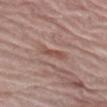Imaged during a routine full-body skin examination; the lesion was not biopsied and no histopathology is available.
On the left thigh.
Automated image analysis of the tile measured a classifier nevus-likeness of about 0/100 and a lesion-detection confidence of about 65/100.
A female patient aged 63 to 67.
The lesion's longest dimension is about 3.5 mm.
The tile uses white-light illumination.
Cropped from a total-body skin-imaging series; the visible field is about 15 mm.